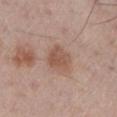{
  "biopsy_status": "not biopsied; imaged during a skin examination",
  "patient": {
    "sex": "male",
    "age_approx": 60
  },
  "lighting": "white-light",
  "site": "left lower leg",
  "lesion_size": {
    "long_diameter_mm_approx": 3.5
  },
  "image": {
    "source": "total-body photography crop",
    "field_of_view_mm": 15
  }
}